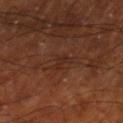- follow-up — catalogued during a skin exam; not biopsied
- location — the right thigh
- size — ~2.5 mm (longest diameter)
- subject — male, aged around 70
- tile lighting — cross-polarized
- image source — 15 mm crop, total-body photography